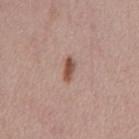Q: Lesion size?
A: ~3 mm (longest diameter)
Q: What kind of image is this?
A: total-body-photography crop, ~15 mm field of view
Q: Lesion location?
A: the chest
Q: Who is the patient?
A: female, aged 63 to 67
Q: Illumination type?
A: white-light